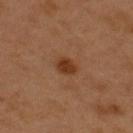• workup: total-body-photography surveillance lesion; no biopsy
• image source: ~15 mm crop, total-body skin-cancer survey
• subject: male, aged approximately 50
• location: the upper back
• TBP lesion metrics: an average lesion color of about L≈36 a*≈23 b*≈33 (CIELAB) and roughly 10 lightness units darker than nearby skin; border irregularity of about 2 on a 0–10 scale, a color-variation rating of about 1.5/10, and radial color variation of about 0.5
• tile lighting: cross-polarized illumination
• lesion diameter: ~2.5 mm (longest diameter)